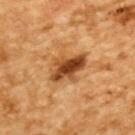subject — male, in their mid-80s or older
tile lighting — cross-polarized illumination
location — the upper back
image — ~15 mm tile from a whole-body skin photo